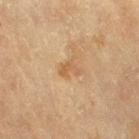* biopsy status · imaged on a skin check; not biopsied
* illumination · cross-polarized
* body site · the right thigh
* diameter · ~2.5 mm (longest diameter)
* patient · female, approximately 80 years of age
* image · total-body-photography crop, ~15 mm field of view